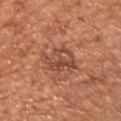• workup · catalogued during a skin exam; not biopsied
• image · 15 mm crop, total-body photography
• lesion size · ~5 mm (longest diameter)
• image-analysis metrics · a lesion color around L≈48 a*≈26 b*≈31 in CIELAB, about 9 CIELAB-L* units darker than the surrounding skin, and a normalized border contrast of about 6.5; border irregularity of about 4.5 on a 0–10 scale, a within-lesion color-variation index near 5/10, and radial color variation of about 2
• anatomic site · the chest
• subject · male, about 65 years old